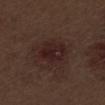  biopsy_status: not biopsied; imaged during a skin examination
  site: leg
  image:
    source: total-body photography crop
    field_of_view_mm: 15
  patient:
    sex: male
    age_approx: 70
  automated_metrics:
    eccentricity: 0.5
    shape_asymmetry: 0.2
    vs_skin_darker_L: 6.0
    vs_skin_contrast_norm: 7.5
  lesion_size:
    long_diameter_mm_approx: 4.0